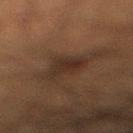notes: no biopsy performed (imaged during a skin exam); subject: male, aged approximately 50; illumination: cross-polarized; imaging modality: ~15 mm tile from a whole-body skin photo; site: the right lower leg.